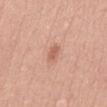Q: Was a biopsy performed?
A: no biopsy performed (imaged during a skin exam)
Q: Where on the body is the lesion?
A: the front of the torso
Q: What are the patient's age and sex?
A: male, approximately 50 years of age
Q: How was this image acquired?
A: total-body-photography crop, ~15 mm field of view